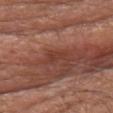Recorded during total-body skin imaging; not selected for excision or biopsy.
A male patient, in their mid-60s.
From the head or neck.
Cropped from a whole-body photographic skin survey; the tile spans about 15 mm.
An algorithmic analysis of the crop reported a shape-asymmetry score of about 0.45 (0 = symmetric). It also reported a classifier nevus-likeness of about 0/100 and lesion-presence confidence of about 100/100.
The tile uses white-light illumination.